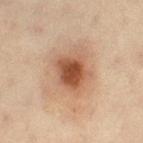Impression: The lesion was photographed on a routine skin check and not biopsied; there is no pathology result. Context: A female subject aged around 45. About 4 mm across. Imaged with cross-polarized lighting. The lesion is on the right thigh. Cropped from a total-body skin-imaging series; the visible field is about 15 mm. An algorithmic analysis of the crop reported a lesion color around L≈44 a*≈21 b*≈29 in CIELAB, roughly 13 lightness units darker than nearby skin, and a lesion-to-skin contrast of about 9.5 (normalized; higher = more distinct). It also reported a border-irregularity rating of about 2/10 and peripheral color asymmetry of about 1.